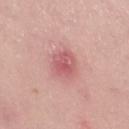This lesion was catalogued during total-body skin photography and was not selected for biopsy. Cropped from a whole-body photographic skin survey; the tile spans about 15 mm. The patient is a female aged approximately 25. The lesion is on the right thigh. Captured under white-light illumination. The total-body-photography lesion software estimated a footprint of about 7 mm² and a symmetry-axis asymmetry near 0.15. The analysis additionally found a within-lesion color-variation index near 4/10 and a peripheral color-asymmetry measure near 1.5. The analysis additionally found a classifier nevus-likeness of about 0/100 and lesion-presence confidence of about 100/100. Approximately 3.5 mm at its widest.